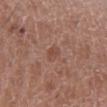Background:
The lesion is located on the left lower leg. A male patient aged approximately 50. This is a white-light tile. A 15 mm crop from a total-body photograph taken for skin-cancer surveillance. The recorded lesion diameter is about 2.5 mm.